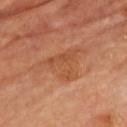follow-up: total-body-photography surveillance lesion; no biopsy
site: the chest
illumination: cross-polarized illumination
TBP lesion metrics: an average lesion color of about L≈49 a*≈25 b*≈36 (CIELAB), about 7 CIELAB-L* units darker than the surrounding skin, and a normalized border contrast of about 5.5
image: total-body-photography crop, ~15 mm field of view
diameter: about 5.5 mm
patient: male, approximately 65 years of age